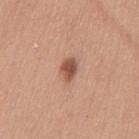Findings:
* follow-up — total-body-photography surveillance lesion; no biopsy
* tile lighting — white-light
* size — about 3 mm
* site — the left thigh
* imaging modality — 15 mm crop, total-body photography
* subject — female, in their 30s